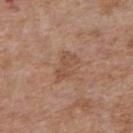<lesion>
  <biopsy_status>not biopsied; imaged during a skin examination</biopsy_status>
  <automated_metrics>
    <area_mm2_approx>5.5</area_mm2_approx>
    <eccentricity>0.85</eccentricity>
    <shape_asymmetry>0.35</shape_asymmetry>
    <border_irregularity_0_10>4.5</border_irregularity_0_10>
    <color_variation_0_10>1.5</color_variation_0_10>
    <peripheral_color_asymmetry>0.5</peripheral_color_asymmetry>
    <lesion_detection_confidence_0_100>100</lesion_detection_confidence_0_100>
  </automated_metrics>
  <image>
    <source>total-body photography crop</source>
    <field_of_view_mm>15</field_of_view_mm>
  </image>
  <site>upper back</site>
  <patient>
    <sex>female</sex>
    <age_approx>75</age_approx>
  </patient>
  <lighting>white-light</lighting>
  <lesion_size>
    <long_diameter_mm_approx>3.5</long_diameter_mm_approx>
  </lesion_size>
</lesion>